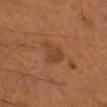Assessment:
No biopsy was performed on this lesion — it was imaged during a full skin examination and was not determined to be concerning.
Context:
This is a cross-polarized tile. Cropped from a whole-body photographic skin survey; the tile spans about 15 mm. Automated image analysis of the tile measured a border-irregularity index near 4/10, a within-lesion color-variation index near 1.5/10, and a peripheral color-asymmetry measure near 0.5. It also reported an automated nevus-likeness rating near 5 out of 100 and a detector confidence of about 100 out of 100 that the crop contains a lesion. On the leg. A male patient, approximately 55 years of age.